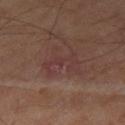Imaged during a routine full-body skin examination; the lesion was not biopsied and no histopathology is available. The lesion's longest dimension is about 4.5 mm. The patient is a male aged 63–67. The tile uses cross-polarized illumination. A 15 mm close-up tile from a total-body photography series done for melanoma screening. Located on the right thigh.